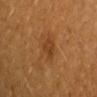Assessment: No biopsy was performed on this lesion — it was imaged during a full skin examination and was not determined to be concerning. Acquisition and patient details: A female patient, aged approximately 30. From the left arm. This image is a 15 mm lesion crop taken from a total-body photograph. Approximately 2.5 mm at its widest.